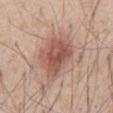Q: What is the anatomic site?
A: the abdomen
Q: How was the tile lit?
A: white-light illumination
Q: What is the lesion's diameter?
A: ≈5.5 mm
Q: What are the patient's age and sex?
A: male, aged approximately 55
Q: What is the imaging modality?
A: ~15 mm tile from a whole-body skin photo